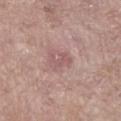The subject is a female aged 83 or older.
From the right lower leg.
A 15 mm close-up extracted from a 3D total-body photography capture.
This is a white-light tile.